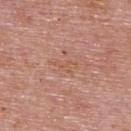biopsy status: total-body-photography surveillance lesion; no biopsy | tile lighting: white-light illumination | image-analysis metrics: a footprint of about 3 mm² and an outline eccentricity of about 0.9 (0 = round, 1 = elongated); a lesion–skin lightness drop of about 5 and a normalized border contrast of about 4.5; a border-irregularity index near 6/10 and internal color variation of about 0 on a 0–10 scale; an automated nevus-likeness rating near 0 out of 100 and a lesion-detection confidence of about 100/100 | acquisition: ~15 mm crop, total-body skin-cancer survey | body site: the upper back | patient: male, aged 73 to 77.